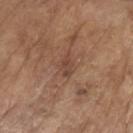No biopsy was performed on this lesion — it was imaged during a full skin examination and was not determined to be concerning. Located on the left upper arm. A region of skin cropped from a whole-body photographic capture, roughly 15 mm wide. The total-body-photography lesion software estimated an area of roughly 3 mm², a shape eccentricity near 0.85, and a symmetry-axis asymmetry near 0.25. It also reported about 7 CIELAB-L* units darker than the surrounding skin and a normalized border contrast of about 6. It also reported a classifier nevus-likeness of about 0/100 and lesion-presence confidence of about 100/100. Longest diameter approximately 3 mm. Imaged with white-light lighting. A male subject, aged 78–82.